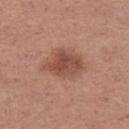{"biopsy_status": "not biopsied; imaged during a skin examination", "image": {"source": "total-body photography crop", "field_of_view_mm": 15}, "automated_metrics": {"cielab_L": 49, "cielab_a": 23, "cielab_b": 29, "vs_skin_darker_L": 10.0, "border_irregularity_0_10": 2.5, "color_variation_0_10": 3.5, "peripheral_color_asymmetry": 1.0}, "patient": {"sex": "female", "age_approx": 30}, "lesion_size": {"long_diameter_mm_approx": 5.0}, "lighting": "white-light", "site": "left thigh"}